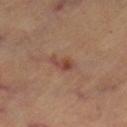<case>
  <biopsy_status>not biopsied; imaged during a skin examination</biopsy_status>
  <lighting>cross-polarized</lighting>
  <patient>
    <age_approx>60</age_approx>
  </patient>
  <image>
    <source>total-body photography crop</source>
    <field_of_view_mm>15</field_of_view_mm>
  </image>
  <automated_metrics>
    <cielab_L>44</cielab_L>
    <cielab_a>22</cielab_a>
    <cielab_b>27</cielab_b>
    <vs_skin_darker_L>8.0</vs_skin_darker_L>
    <border_irregularity_0_10>4.5</border_irregularity_0_10>
    <color_variation_0_10>3.0</color_variation_0_10>
    <peripheral_color_asymmetry>1.0</peripheral_color_asymmetry>
  </automated_metrics>
  <site>left thigh</site>
</case>